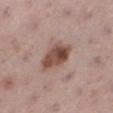The lesion was tiled from a total-body skin photograph and was not biopsied.
Automated tile analysis of the lesion measured a lesion color around L≈48 a*≈20 b*≈25 in CIELAB and a normalized border contrast of about 10. And it measured a border-irregularity rating of about 2/10 and internal color variation of about 6.5 on a 0–10 scale.
Measured at roughly 4.5 mm in maximum diameter.
This image is a 15 mm lesion crop taken from a total-body photograph.
The tile uses white-light illumination.
A female subject in their mid- to late 50s.
The lesion is located on the left lower leg.